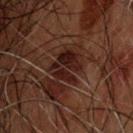Q: What lighting was used for the tile?
A: cross-polarized illumination
Q: Lesion location?
A: the head or neck
Q: What kind of image is this?
A: ~15 mm tile from a whole-body skin photo
Q: Who is the patient?
A: male, approximately 50 years of age